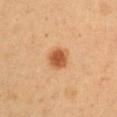The lesion is located on the right upper arm. Cropped from a whole-body photographic skin survey; the tile spans about 15 mm. Approximately 3 mm at its widest. A male subject, roughly 55 years of age. The tile uses cross-polarized illumination.